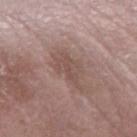Q: Was a biopsy performed?
A: catalogued during a skin exam; not biopsied
Q: What kind of image is this?
A: ~15 mm crop, total-body skin-cancer survey
Q: Who is the patient?
A: male, in their mid-70s
Q: What lighting was used for the tile?
A: white-light illumination
Q: Where on the body is the lesion?
A: the arm
Q: Automated lesion metrics?
A: a lesion area of about 2 mm², an eccentricity of roughly 0.95, and two-axis asymmetry of about 0.55; an average lesion color of about L≈48 a*≈17 b*≈23 (CIELAB), a lesion–skin lightness drop of about 6, and a normalized border contrast of about 5; a color-variation rating of about 0/10 and a peripheral color-asymmetry measure near 0
Q: How large is the lesion?
A: ≈2.5 mm